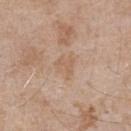Q: Was a biopsy performed?
A: catalogued during a skin exam; not biopsied
Q: How large is the lesion?
A: ~3 mm (longest diameter)
Q: Patient demographics?
A: male, in their mid-60s
Q: Automated lesion metrics?
A: a detector confidence of about 100 out of 100 that the crop contains a lesion
Q: How was this image acquired?
A: ~15 mm crop, total-body skin-cancer survey
Q: Where on the body is the lesion?
A: the chest